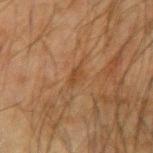The lesion was photographed on a routine skin check and not biopsied; there is no pathology result. The subject is a male aged approximately 65. A roughly 15 mm field-of-view crop from a total-body skin photograph. The recorded lesion diameter is about 2.5 mm. An algorithmic analysis of the crop reported a footprint of about 2.5 mm², a shape eccentricity near 0.85, and a symmetry-axis asymmetry near 0.25. The analysis additionally found border irregularity of about 3 on a 0–10 scale, a within-lesion color-variation index near 1/10, and radial color variation of about 0.5. And it measured an automated nevus-likeness rating near 0 out of 100 and a detector confidence of about 100 out of 100 that the crop contains a lesion. This is a cross-polarized tile. The lesion is on the left forearm.